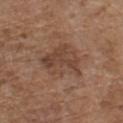Clinical impression:
No biopsy was performed on this lesion — it was imaged during a full skin examination and was not determined to be concerning.
Acquisition and patient details:
The lesion's longest dimension is about 5 mm. The lesion is on the front of the torso. A lesion tile, about 15 mm wide, cut from a 3D total-body photograph. A male subject roughly 75 years of age.